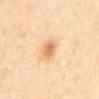Imaged during a routine full-body skin examination; the lesion was not biopsied and no histopathology is available.
This is a cross-polarized tile.
A male subject, aged 63–67.
A 15 mm crop from a total-body photograph taken for skin-cancer surveillance.
Automated tile analysis of the lesion measured an area of roughly 4.5 mm², an outline eccentricity of about 0.7 (0 = round, 1 = elongated), and a symmetry-axis asymmetry near 0.2. And it measured an average lesion color of about L≈71 a*≈21 b*≈40 (CIELAB). It also reported a border-irregularity index near 2/10. It also reported a lesion-detection confidence of about 100/100.
The lesion is located on the mid back.